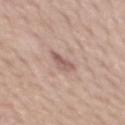Clinical impression: The lesion was tiled from a total-body skin photograph and was not biopsied. Image and clinical context: The lesion's longest dimension is about 3.5 mm. A 15 mm crop from a total-body photograph taken for skin-cancer surveillance. A male subject, aged 48–52. Imaged with white-light lighting. The lesion-visualizer software estimated an average lesion color of about L≈58 a*≈19 b*≈23 (CIELAB) and a normalized lesion–skin contrast near 7. The analysis additionally found internal color variation of about 2 on a 0–10 scale and a peripheral color-asymmetry measure near 0.5. The software also gave a nevus-likeness score of about 0/100 and a lesion-detection confidence of about 95/100. On the mid back.